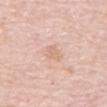This lesion was catalogued during total-body skin photography and was not selected for biopsy.
Imaged with white-light lighting.
A close-up tile cropped from a whole-body skin photograph, about 15 mm across.
The patient is a male approximately 80 years of age.
Located on the mid back.